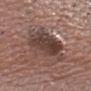| field | value |
|---|---|
| workup | catalogued during a skin exam; not biopsied |
| imaging modality | 15 mm crop, total-body photography |
| anatomic site | the head or neck |
| subject | male, in their mid- to late 50s |
| diameter | about 5.5 mm |
| tile lighting | white-light |